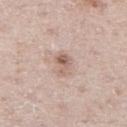This lesion was catalogued during total-body skin photography and was not selected for biopsy. A roughly 15 mm field-of-view crop from a total-body skin photograph. A male patient, in their mid- to late 60s. Longest diameter approximately 3 mm. The total-body-photography lesion software estimated a lesion color around L≈60 a*≈17 b*≈25 in CIELAB and a normalized border contrast of about 7. The analysis additionally found border irregularity of about 3.5 on a 0–10 scale and a color-variation rating of about 7.5/10. From the right thigh.